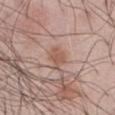<lesion>
<biopsy_status>not biopsied; imaged during a skin examination</biopsy_status>
<lighting>white-light</lighting>
<image>
  <source>total-body photography crop</source>
  <field_of_view_mm>15</field_of_view_mm>
</image>
<automated_metrics>
  <area_mm2_approx>4.0</area_mm2_approx>
  <eccentricity>0.75</eccentricity>
  <shape_asymmetry>0.25</shape_asymmetry>
  <cielab_L>55</cielab_L>
  <cielab_a>20</cielab_a>
  <cielab_b>27</cielab_b>
  <nevus_likeness_0_100>45</nevus_likeness_0_100>
</automated_metrics>
<patient>
  <sex>male</sex>
  <age_approx>55</age_approx>
</patient>
<site>abdomen</site>
<lesion_size>
  <long_diameter_mm_approx>3.0</long_diameter_mm_approx>
</lesion_size>
</lesion>